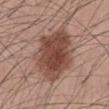A male patient, aged approximately 40. On the abdomen. A 15 mm close-up tile from a total-body photography series done for melanoma screening. Captured under white-light illumination. The lesion-visualizer software estimated a lesion color around L≈46 a*≈21 b*≈26 in CIELAB, a lesion–skin lightness drop of about 14, and a normalized border contrast of about 10.